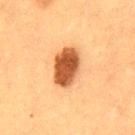An algorithmic analysis of the crop reported an eccentricity of roughly 0.75 and a symmetry-axis asymmetry near 0.15. The analysis additionally found a classifier nevus-likeness of about 100/100 and a lesion-detection confidence of about 100/100. A lesion tile, about 15 mm wide, cut from a 3D total-body photograph. Captured under cross-polarized illumination. A male subject, aged 48–52. Located on the mid back. Measured at roughly 4.5 mm in maximum diameter.